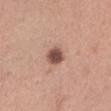Recorded during total-body skin imaging; not selected for excision or biopsy. A female patient aged 28 to 32. About 3 mm across. A 15 mm close-up extracted from a 3D total-body photography capture. From the left thigh. Imaged with white-light lighting.